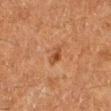patient = female, about 50 years old | location = the right lower leg | automated lesion analysis = a lesion color around L≈40 a*≈22 b*≈31 in CIELAB and a normalized border contrast of about 7; a border-irregularity index near 3.5/10, a color-variation rating of about 2.5/10, and radial color variation of about 0.5; a nevus-likeness score of about 65/100 and a lesion-detection confidence of about 100/100 | tile lighting = cross-polarized | imaging modality = total-body-photography crop, ~15 mm field of view | lesion diameter = ~2.5 mm (longest diameter).